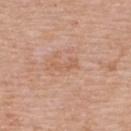{
  "biopsy_status": "not biopsied; imaged during a skin examination",
  "image": {
    "source": "total-body photography crop",
    "field_of_view_mm": 15
  },
  "site": "upper back",
  "lighting": "white-light",
  "lesion_size": {
    "long_diameter_mm_approx": 3.0
  },
  "patient": {
    "sex": "female",
    "age_approx": 65
  },
  "automated_metrics": {
    "area_mm2_approx": 3.5,
    "eccentricity": 0.9,
    "cielab_L": 60,
    "cielab_a": 21,
    "cielab_b": 32,
    "vs_skin_darker_L": 6.0,
    "vs_skin_contrast_norm": 5.0,
    "border_irregularity_0_10": 5.0,
    "color_variation_0_10": 0.0,
    "peripheral_color_asymmetry": 0.0
  }
}